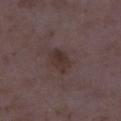Q: How was the tile lit?
A: white-light illumination
Q: What are the patient's age and sex?
A: female, roughly 35 years of age
Q: Lesion location?
A: the right lower leg
Q: What is the lesion's diameter?
A: about 3.5 mm
Q: How was this image acquired?
A: ~15 mm tile from a whole-body skin photo